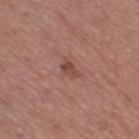Captured during whole-body skin photography for melanoma surveillance; the lesion was not biopsied.
The lesion is on the right thigh.
The recorded lesion diameter is about 2.5 mm.
A female subject, aged 58–62.
Captured under white-light illumination.
A region of skin cropped from a whole-body photographic capture, roughly 15 mm wide.